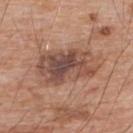Assessment:
Recorded during total-body skin imaging; not selected for excision or biopsy.